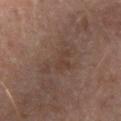  biopsy_status: not biopsied; imaged during a skin examination
  site: right upper arm
  image:
    source: total-body photography crop
    field_of_view_mm: 15
  patient:
    sex: male
    age_approx: 60
  lighting: cross-polarized
  lesion_size:
    long_diameter_mm_approx: 6.0